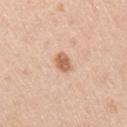Q: Was a biopsy performed?
A: total-body-photography surveillance lesion; no biopsy
Q: What kind of image is this?
A: ~15 mm crop, total-body skin-cancer survey
Q: How was the tile lit?
A: white-light illumination
Q: Automated lesion metrics?
A: an automated nevus-likeness rating near 95 out of 100 and a lesion-detection confidence of about 100/100
Q: What is the anatomic site?
A: the right upper arm
Q: What are the patient's age and sex?
A: male, aged 43 to 47